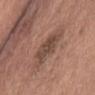biopsy status = catalogued during a skin exam; not biopsied | tile lighting = white-light | patient = female, about 55 years old | acquisition = ~15 mm crop, total-body skin-cancer survey | diameter = about 6 mm | location = the abdomen.